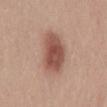The lesion was tiled from a total-body skin photograph and was not biopsied. The lesion-visualizer software estimated a border-irregularity index near 2/10, internal color variation of about 4 on a 0–10 scale, and peripheral color asymmetry of about 1. It also reported a lesion-detection confidence of about 100/100. This is a white-light tile. A close-up tile cropped from a whole-body skin photograph, about 15 mm across. The subject is a male aged 28 to 32.